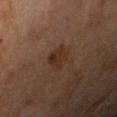Assessment:
Captured during whole-body skin photography for melanoma surveillance; the lesion was not biopsied.
Background:
Approximately 3 mm at its widest. A 15 mm close-up extracted from a 3D total-body photography capture. Located on the right upper arm. A female subject, aged 78 to 82. This is a cross-polarized tile.